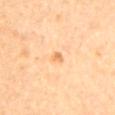Part of a total-body skin-imaging series; this lesion was reviewed on a skin check and was not flagged for biopsy. Imaged with cross-polarized lighting. A male subject about 60 years old. A lesion tile, about 15 mm wide, cut from a 3D total-body photograph. On the mid back. Measured at roughly 1.5 mm in maximum diameter.